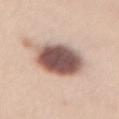notes: imaged on a skin check; not biopsied | site: the abdomen | image: 15 mm crop, total-body photography | automated metrics: a footprint of about 20 mm², an eccentricity of roughly 0.65, and a shape-asymmetry score of about 0.15 (0 = symmetric); a border-irregularity rating of about 1.5/10, a color-variation rating of about 6.5/10, and peripheral color asymmetry of about 1.5; a classifier nevus-likeness of about 35/100 and a detector confidence of about 100 out of 100 that the crop contains a lesion | size: ~6 mm (longest diameter) | subject: female, approximately 45 years of age.